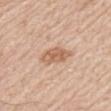{"biopsy_status": "not biopsied; imaged during a skin examination", "lesion_size": {"long_diameter_mm_approx": 3.5}, "patient": {"sex": "male", "age_approx": 80}, "site": "left upper arm", "image": {"source": "total-body photography crop", "field_of_view_mm": 15}, "automated_metrics": {"eccentricity": 0.7, "shape_asymmetry": 0.2, "cielab_L": 62, "cielab_a": 20, "cielab_b": 32, "vs_skin_darker_L": 10.0, "vs_skin_contrast_norm": 7.0, "lesion_detection_confidence_0_100": 100}, "lighting": "white-light"}